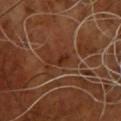Captured during whole-body skin photography for melanoma surveillance; the lesion was not biopsied.
Cropped from a total-body skin-imaging series; the visible field is about 15 mm.
From the chest.
The patient is a male approximately 65 years of age.
Measured at roughly 3 mm in maximum diameter.